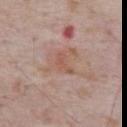notes = total-body-photography surveillance lesion; no biopsy
anatomic site = the front of the torso
size = about 3 mm
imaging modality = 15 mm crop, total-body photography
subject = male, aged approximately 70